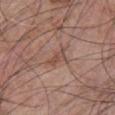Assessment: Imaged during a routine full-body skin examination; the lesion was not biopsied and no histopathology is available. Image and clinical context: The subject is a male approximately 70 years of age. Cropped from a whole-body photographic skin survey; the tile spans about 15 mm. Located on the chest. The lesion-visualizer software estimated a footprint of about 3.5 mm² and a symmetry-axis asymmetry near 0.5. It also reported about 7 CIELAB-L* units darker than the surrounding skin and a normalized border contrast of about 5.5. The analysis additionally found a nevus-likeness score of about 0/100 and a lesion-detection confidence of about 95/100.